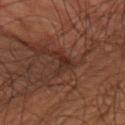Imaged during a routine full-body skin examination; the lesion was not biopsied and no histopathology is available.
From the right upper arm.
A male patient, aged 68–72.
The lesion's longest dimension is about 3.5 mm.
A 15 mm close-up extracted from a 3D total-body photography capture.
Captured under cross-polarized illumination.